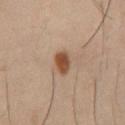Impression:
Part of a total-body skin-imaging series; this lesion was reviewed on a skin check and was not flagged for biopsy.
Clinical summary:
A close-up tile cropped from a whole-body skin photograph, about 15 mm across. Located on the chest. Measured at roughly 3 mm in maximum diameter. The lesion-visualizer software estimated a lesion area of about 4.5 mm² and an eccentricity of roughly 0.8. It also reported a mean CIELAB color near L≈47 a*≈20 b*≈31 and a lesion-to-skin contrast of about 10.5 (normalized; higher = more distinct). The software also gave a color-variation rating of about 2/10 and radial color variation of about 0.5. The software also gave a nevus-likeness score of about 100/100 and lesion-presence confidence of about 100/100. The subject is a male about 40 years old. Captured under cross-polarized illumination.